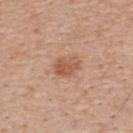Q: Is there a histopathology result?
A: catalogued during a skin exam; not biopsied
Q: Lesion size?
A: ≈3.5 mm
Q: Illumination type?
A: white-light illumination
Q: What are the patient's age and sex?
A: male, aged around 55
Q: Automated lesion metrics?
A: an area of roughly 7 mm², an eccentricity of roughly 0.65, and a shape-asymmetry score of about 0.15 (0 = symmetric); about 9 CIELAB-L* units darker than the surrounding skin and a normalized border contrast of about 6.5; a border-irregularity rating of about 1.5/10, a within-lesion color-variation index near 3.5/10, and a peripheral color-asymmetry measure near 1.5
Q: What kind of image is this?
A: total-body-photography crop, ~15 mm field of view
Q: Lesion location?
A: the upper back